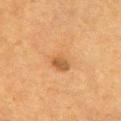Q: What is the anatomic site?
A: the chest
Q: How large is the lesion?
A: ≈3.5 mm
Q: Who is the patient?
A: female, approximately 55 years of age
Q: How was the tile lit?
A: cross-polarized illumination
Q: What kind of image is this?
A: 15 mm crop, total-body photography